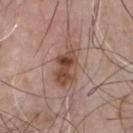From the chest.
This image is a 15 mm lesion crop taken from a total-body photograph.
The lesion's longest dimension is about 6 mm.
This is a white-light tile.
The subject is a male aged 53–57.